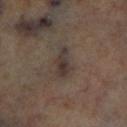{
  "biopsy_status": "not biopsied; imaged during a skin examination",
  "image": {
    "source": "total-body photography crop",
    "field_of_view_mm": 15
  },
  "site": "right lower leg",
  "automated_metrics": {
    "cielab_L": 34,
    "cielab_a": 11,
    "cielab_b": 18,
    "vs_skin_darker_L": 8.0,
    "vs_skin_contrast_norm": 8.5,
    "border_irregularity_0_10": 3.5,
    "color_variation_0_10": 4.5,
    "peripheral_color_asymmetry": 1.5,
    "nevus_likeness_0_100": 0
  },
  "lesion_size": {
    "long_diameter_mm_approx": 4.0
  }
}